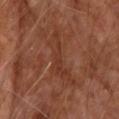Context:
Automated tile analysis of the lesion measured a mean CIELAB color near L≈35 a*≈24 b*≈29, about 5 CIELAB-L* units darker than the surrounding skin, and a lesion-to-skin contrast of about 5 (normalized; higher = more distinct). The analysis additionally found a border-irregularity index near 8.5/10, a color-variation rating of about 2.5/10, and a peripheral color-asymmetry measure near 1. Located on the upper back. Imaged with cross-polarized lighting. The patient is a male aged 68–72. This image is a 15 mm lesion crop taken from a total-body photograph. About 7 mm across.